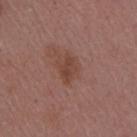notes: catalogued during a skin exam; not biopsied
subject: female, aged 43 to 47
image: ~15 mm crop, total-body skin-cancer survey
body site: the arm
illumination: white-light
lesion size: ≈3 mm
image-analysis metrics: border irregularity of about 2.5 on a 0–10 scale and radial color variation of about 1